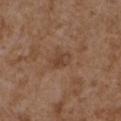Context: This is a white-light tile. This image is a 15 mm lesion crop taken from a total-body photograph. An algorithmic analysis of the crop reported a nevus-likeness score of about 0/100 and a lesion-detection confidence of about 100/100. Longest diameter approximately 3 mm. From the right forearm. The patient is a female aged 33–37.